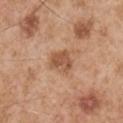follow-up: imaged on a skin check; not biopsied | lesion diameter: ≈3 mm | image: 15 mm crop, total-body photography | location: the right upper arm | automated metrics: an area of roughly 6 mm², an eccentricity of roughly 0.45, and a shape-asymmetry score of about 0.3 (0 = symmetric); a mean CIELAB color near L≈53 a*≈22 b*≈33, roughly 10 lightness units darker than nearby skin, and a normalized lesion–skin contrast near 7.5; peripheral color asymmetry of about 1; a classifier nevus-likeness of about 20/100 and a lesion-detection confidence of about 100/100 | patient: male, aged 53 to 57 | tile lighting: white-light.